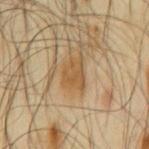Assessment: No biopsy was performed on this lesion — it was imaged during a full skin examination and was not determined to be concerning. Background: Cropped from a whole-body photographic skin survey; the tile spans about 15 mm. The lesion is located on the mid back. The subject is a male aged approximately 65.